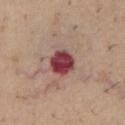Part of a total-body skin-imaging series; this lesion was reviewed on a skin check and was not flagged for biopsy. This is a white-light tile. A male patient, aged 58–62. The lesion-visualizer software estimated a footprint of about 8.5 mm² and two-axis asymmetry of about 0.1. The software also gave a lesion color around L≈42 a*≈30 b*≈20 in CIELAB, a lesion–skin lightness drop of about 18, and a lesion-to-skin contrast of about 13.5 (normalized; higher = more distinct). The analysis additionally found border irregularity of about 1 on a 0–10 scale, internal color variation of about 5.5 on a 0–10 scale, and peripheral color asymmetry of about 2. From the chest. A roughly 15 mm field-of-view crop from a total-body skin photograph.